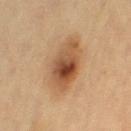Impression: No biopsy was performed on this lesion — it was imaged during a full skin examination and was not determined to be concerning. Image and clinical context: From the leg. A 15 mm close-up extracted from a 3D total-body photography capture. The patient is a female in their 40s.